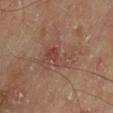Clinical impression: No biopsy was performed on this lesion — it was imaged during a full skin examination and was not determined to be concerning. Image and clinical context: A 15 mm close-up extracted from a 3D total-body photography capture. The lesion's longest dimension is about 7 mm. The lesion-visualizer software estimated a footprint of about 17 mm², an outline eccentricity of about 0.8 (0 = round, 1 = elongated), and a shape-asymmetry score of about 0.5 (0 = symmetric). It also reported a lesion color around L≈46 a*≈19 b*≈25 in CIELAB, about 6 CIELAB-L* units darker than the surrounding skin, and a normalized lesion–skin contrast near 5.5. The lesion is located on the leg. Imaged with cross-polarized lighting. A male patient, approximately 65 years of age.